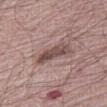This lesion was catalogued during total-body skin photography and was not selected for biopsy. Captured under white-light illumination. On the right thigh. A male patient, about 65 years old. A 15 mm close-up tile from a total-body photography series done for melanoma screening. The lesion-visualizer software estimated a shape eccentricity near 0.9. The software also gave an average lesion color of about L≈48 a*≈18 b*≈20 (CIELAB), a lesion–skin lightness drop of about 12, and a lesion-to-skin contrast of about 8.5 (normalized; higher = more distinct). About 4.5 mm across.